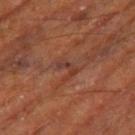- follow-up · imaged on a skin check; not biopsied
- imaging modality · ~15 mm crop, total-body skin-cancer survey
- anatomic site · the leg
- subject · male, in their mid- to late 60s
- automated lesion analysis · a shape eccentricity near 0.85 and a symmetry-axis asymmetry near 0.45; a lesion color around L≈35 a*≈23 b*≈26 in CIELAB and roughly 7 lightness units darker than nearby skin; a detector confidence of about 55 out of 100 that the crop contains a lesion
- lighting · cross-polarized illumination
- diameter · ~2.5 mm (longest diameter)